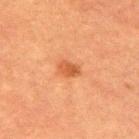biopsy status: imaged on a skin check; not biopsied | lesion diameter: about 2.5 mm | automated lesion analysis: an outline eccentricity of about 0.75 (0 = round, 1 = elongated); a mean CIELAB color near L≈45 a*≈25 b*≈36; peripheral color asymmetry of about 1; a lesion-detection confidence of about 100/100 | acquisition: 15 mm crop, total-body photography | tile lighting: cross-polarized | location: the upper back | subject: male, approximately 50 years of age.